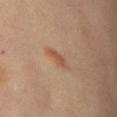Impression: Part of a total-body skin-imaging series; this lesion was reviewed on a skin check and was not flagged for biopsy. Context: The tile uses cross-polarized illumination. A close-up tile cropped from a whole-body skin photograph, about 15 mm across. The lesion is located on the right upper arm. A female patient aged around 55. The lesion-visualizer software estimated a lesion area of about 3.5 mm², an outline eccentricity of about 0.85 (0 = round, 1 = elongated), and a shape-asymmetry score of about 0.3 (0 = symmetric). The analysis additionally found a detector confidence of about 100 out of 100 that the crop contains a lesion.